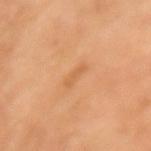  biopsy_status: not biopsied; imaged during a skin examination
  lighting: cross-polarized
  patient:
    sex: female
    age_approx: 70
  image:
    source: total-body photography crop
    field_of_view_mm: 15
  lesion_size:
    long_diameter_mm_approx: 2.5
  site: arm